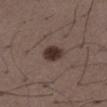– lighting — white-light illumination
– patient — male, approximately 50 years of age
– acquisition — ~15 mm crop, total-body skin-cancer survey
– lesion size — ~3 mm (longest diameter)
– anatomic site — the left thigh